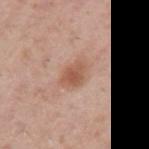Impression:
Recorded during total-body skin imaging; not selected for excision or biopsy.
Background:
A roughly 15 mm field-of-view crop from a total-body skin photograph. The lesion is on the left upper arm. The lesion's longest dimension is about 3.5 mm. A male patient, about 40 years old. The total-body-photography lesion software estimated a lesion color around L≈56 a*≈22 b*≈30 in CIELAB, about 10 CIELAB-L* units darker than the surrounding skin, and a lesion-to-skin contrast of about 7 (normalized; higher = more distinct). It also reported a classifier nevus-likeness of about 85/100 and lesion-presence confidence of about 100/100.